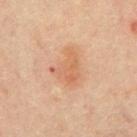Part of a total-body skin-imaging series; this lesion was reviewed on a skin check and was not flagged for biopsy. Longest diameter approximately 4.5 mm. A male patient aged approximately 65. This is a cross-polarized tile. Located on the abdomen. A 15 mm crop from a total-body photograph taken for skin-cancer surveillance.